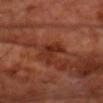No biopsy was performed on this lesion — it was imaged during a full skin examination and was not determined to be concerning. A male patient in their 70s. The lesion's longest dimension is about 3.5 mm. The lesion is located on the chest. Imaged with cross-polarized lighting. This image is a 15 mm lesion crop taken from a total-body photograph. Automated image analysis of the tile measured an outline eccentricity of about 0.8 (0 = round, 1 = elongated) and a shape-asymmetry score of about 0.5 (0 = symmetric). The analysis additionally found about 7 CIELAB-L* units darker than the surrounding skin and a normalized lesion–skin contrast near 7. The software also gave a border-irregularity rating of about 5.5/10. It also reported lesion-presence confidence of about 95/100.